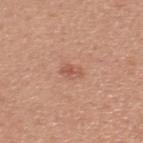This lesion was catalogued during total-body skin photography and was not selected for biopsy.
A male subject, about 50 years old.
The lesion is on the upper back.
The tile uses white-light illumination.
Approximately 2.5 mm at its widest.
A lesion tile, about 15 mm wide, cut from a 3D total-body photograph.